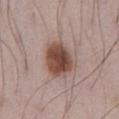biopsy status=total-body-photography surveillance lesion; no biopsy
imaging modality=total-body-photography crop, ~15 mm field of view
site=the front of the torso
illumination=white-light illumination
patient=male, aged 68 to 72
diameter=~5 mm (longest diameter)